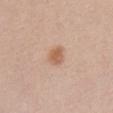Impression:
Recorded during total-body skin imaging; not selected for excision or biopsy.
Background:
About 2.5 mm across. From the chest. Cropped from a whole-body photographic skin survey; the tile spans about 15 mm. The patient is a female aged 28–32. Imaged with white-light lighting.